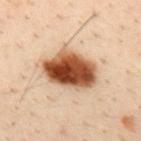Q: Was a biopsy performed?
A: no biopsy performed (imaged during a skin exam)
Q: Patient demographics?
A: male, in their 30s
Q: How was the tile lit?
A: cross-polarized illumination
Q: How was this image acquired?
A: total-body-photography crop, ~15 mm field of view
Q: What did automated image analysis measure?
A: a footprint of about 20 mm², an outline eccentricity of about 0.75 (0 = round, 1 = elongated), and a shape-asymmetry score of about 0.2 (0 = symmetric); a border-irregularity index near 2/10 and radial color variation of about 3.5
Q: What is the anatomic site?
A: the mid back
Q: What is the lesion's diameter?
A: ~6.5 mm (longest diameter)